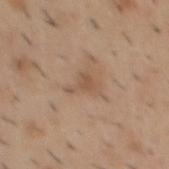tile lighting: white-light
location: the mid back
imaging modality: 15 mm crop, total-body photography
automated lesion analysis: an average lesion color of about L≈53 a*≈17 b*≈31 (CIELAB), roughly 7 lightness units darker than nearby skin, and a normalized border contrast of about 5.5; border irregularity of about 6.5 on a 0–10 scale, internal color variation of about 1.5 on a 0–10 scale, and radial color variation of about 0.5
subject: male, aged 38 to 42